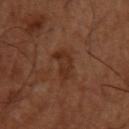This lesion was catalogued during total-body skin photography and was not selected for biopsy. Longest diameter approximately 4.5 mm. The tile uses cross-polarized illumination. The lesion is on the left upper arm. A roughly 15 mm field-of-view crop from a total-body skin photograph. A male patient in their 50s.